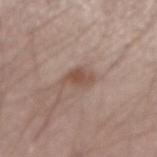Q: Is there a histopathology result?
A: no biopsy performed (imaged during a skin exam)
Q: Patient demographics?
A: male, roughly 45 years of age
Q: Lesion location?
A: the left forearm
Q: What is the imaging modality?
A: 15 mm crop, total-body photography
Q: What lighting was used for the tile?
A: white-light illumination